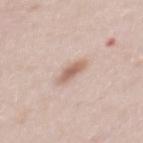<record>
  <biopsy_status>not biopsied; imaged during a skin examination</biopsy_status>
  <patient>
    <sex>female</sex>
    <age_approx>40</age_approx>
  </patient>
  <image>
    <source>total-body photography crop</source>
    <field_of_view_mm>15</field_of_view_mm>
  </image>
  <lesion_size>
    <long_diameter_mm_approx>3.0</long_diameter_mm_approx>
  </lesion_size>
  <site>mid back</site>
  <lighting>white-light</lighting>
  <automated_metrics>
    <peripheral_color_asymmetry>0.5</peripheral_color_asymmetry>
    <nevus_likeness_0_100>75</nevus_likeness_0_100>
    <lesion_detection_confidence_0_100>100</lesion_detection_confidence_0_100>
  </automated_metrics>
</record>